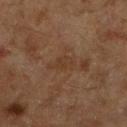Q: Is there a histopathology result?
A: no biopsy performed (imaged during a skin exam)
Q: What is the lesion's diameter?
A: ≈3 mm
Q: What are the patient's age and sex?
A: male, aged 58–62
Q: How was this image acquired?
A: ~15 mm tile from a whole-body skin photo
Q: Lesion location?
A: the left lower leg
Q: How was the tile lit?
A: cross-polarized illumination